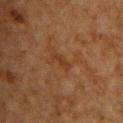The lesion was tiled from a total-body skin photograph and was not biopsied. This image is a 15 mm lesion crop taken from a total-body photograph. The lesion is located on the chest. About 3 mm across. A male subject aged 58 to 62. The tile uses cross-polarized illumination.